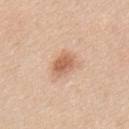Context: From the mid back. This is a white-light tile. Automated tile analysis of the lesion measured an outline eccentricity of about 0.75 (0 = round, 1 = elongated) and two-axis asymmetry of about 0.2. It also reported a mean CIELAB color near L≈63 a*≈21 b*≈33, a lesion–skin lightness drop of about 11, and a normalized border contrast of about 7.5. It also reported a classifier nevus-likeness of about 95/100 and a detector confidence of about 100 out of 100 that the crop contains a lesion. The recorded lesion diameter is about 3.5 mm. A 15 mm close-up extracted from a 3D total-body photography capture. A male patient, in their mid- to late 30s.